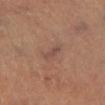biopsy status: total-body-photography surveillance lesion; no biopsy | site: the left lower leg | automated metrics: a shape eccentricity near 0.95 and two-axis asymmetry of about 0.4; a mean CIELAB color near L≈44 a*≈19 b*≈23, roughly 7 lightness units darker than nearby skin, and a lesion-to-skin contrast of about 6.5 (normalized; higher = more distinct) | illumination: cross-polarized | patient: female, aged around 65 | lesion size: about 3 mm | image source: total-body-photography crop, ~15 mm field of view.